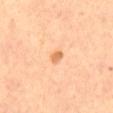Cropped from a whole-body photographic skin survey; the tile spans about 15 mm. Located on the front of the torso. Longest diameter approximately 2 mm. The tile uses cross-polarized illumination. The lesion-visualizer software estimated an area of roughly 2 mm², a shape eccentricity near 0.65, and two-axis asymmetry of about 0.15. The analysis additionally found a lesion color around L≈65 a*≈25 b*≈41 in CIELAB and a lesion-to-skin contrast of about 7.5 (normalized; higher = more distinct). The analysis additionally found a border-irregularity index near 1.5/10, a color-variation rating of about 1/10, and a peripheral color-asymmetry measure near 0.5. A female patient aged around 65.